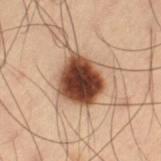Impression:
Imaged during a routine full-body skin examination; the lesion was not biopsied and no histopathology is available.
Clinical summary:
Longest diameter approximately 5 mm. Located on the left thigh. A 15 mm close-up tile from a total-body photography series done for melanoma screening. Imaged with cross-polarized lighting. The lesion-visualizer software estimated an area of roughly 18 mm², an outline eccentricity of about 0.4 (0 = round, 1 = elongated), and a symmetry-axis asymmetry near 0.15. The analysis additionally found border irregularity of about 1.5 on a 0–10 scale, internal color variation of about 6.5 on a 0–10 scale, and a peripheral color-asymmetry measure near 1.5. The software also gave a classifier nevus-likeness of about 100/100 and a detector confidence of about 100 out of 100 that the crop contains a lesion. A male patient, aged around 55.